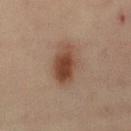Imaged during a routine full-body skin examination; the lesion was not biopsied and no histopathology is available. Approximately 5 mm at its widest. The lesion is located on the right thigh. The patient is a female aged approximately 45. This is a cross-polarized tile. Cropped from a total-body skin-imaging series; the visible field is about 15 mm.